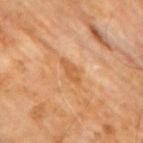Clinical impression:
This lesion was catalogued during total-body skin photography and was not selected for biopsy.
Background:
A male patient, roughly 60 years of age. A lesion tile, about 15 mm wide, cut from a 3D total-body photograph. Located on the arm. Longest diameter approximately 3.5 mm.